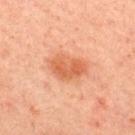Captured during whole-body skin photography for melanoma surveillance; the lesion was not biopsied. The tile uses cross-polarized illumination. A male subject, aged around 30. About 4.5 mm across. Cropped from a whole-body photographic skin survey; the tile spans about 15 mm. The lesion is on the upper back.